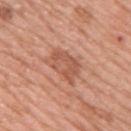Clinical summary: The lesion's longest dimension is about 4.5 mm. The tile uses white-light illumination. Located on the right upper arm. A female patient, in their mid-40s. Automated image analysis of the tile measured a shape eccentricity near 0.8 and a symmetry-axis asymmetry near 0.3. The analysis additionally found an average lesion color of about L≈55 a*≈25 b*≈32 (CIELAB), roughly 9 lightness units darker than nearby skin, and a normalized lesion–skin contrast near 6. It also reported a border-irregularity rating of about 3/10. The analysis additionally found an automated nevus-likeness rating near 15 out of 100 and lesion-presence confidence of about 100/100. A region of skin cropped from a whole-body photographic capture, roughly 15 mm wide.